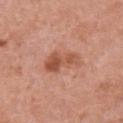Q: Was a biopsy performed?
A: imaged on a skin check; not biopsied
Q: Where on the body is the lesion?
A: the chest
Q: What is the imaging modality?
A: ~15 mm tile from a whole-body skin photo
Q: What lighting was used for the tile?
A: white-light
Q: Who is the patient?
A: female, in their 40s
Q: How large is the lesion?
A: ~4.5 mm (longest diameter)